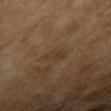* workup: imaged on a skin check; not biopsied
* anatomic site: the right upper arm
* patient: female, in their 60s
* imaging modality: ~15 mm crop, total-body skin-cancer survey